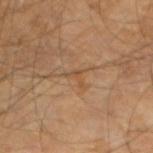lesion size: ~3 mm (longest diameter) | body site: the right arm | automated lesion analysis: a shape eccentricity near 0.8 and a shape-asymmetry score of about 0.8 (0 = symmetric) | imaging modality: total-body-photography crop, ~15 mm field of view | subject: male, approximately 60 years of age.